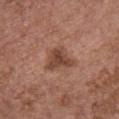| feature | finding |
|---|---|
| notes | no biopsy performed (imaged during a skin exam) |
| patient | female, approximately 65 years of age |
| body site | the chest |
| TBP lesion metrics | an average lesion color of about L≈45 a*≈22 b*≈28 (CIELAB) and a normalized border contrast of about 8; border irregularity of about 4.5 on a 0–10 scale, a color-variation rating of about 3.5/10, and peripheral color asymmetry of about 1 |
| acquisition | 15 mm crop, total-body photography |